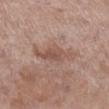No biopsy was performed on this lesion — it was imaged during a full skin examination and was not determined to be concerning.
The total-body-photography lesion software estimated a lesion area of about 10 mm², an outline eccentricity of about 0.9 (0 = round, 1 = elongated), and a shape-asymmetry score of about 0.3 (0 = symmetric). And it measured border irregularity of about 4.5 on a 0–10 scale and radial color variation of about 1. The software also gave an automated nevus-likeness rating near 0 out of 100 and a lesion-detection confidence of about 100/100.
A 15 mm close-up tile from a total-body photography series done for melanoma screening.
A female patient approximately 50 years of age.
From the right lower leg.
The lesion's longest dimension is about 5.5 mm.
Imaged with white-light lighting.